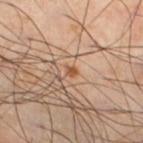biopsy status: catalogued during a skin exam; not biopsied
diameter: about 1.5 mm
image: 15 mm crop, total-body photography
subject: male, aged around 40
lighting: cross-polarized
automated lesion analysis: an eccentricity of roughly 0.6; a mean CIELAB color near L≈53 a*≈23 b*≈36, about 10 CIELAB-L* units darker than the surrounding skin, and a normalized lesion–skin contrast near 8.5; a border-irregularity rating of about 2.5/10, a within-lesion color-variation index near 0/10, and a peripheral color-asymmetry measure near 0
location: the left lower leg